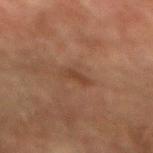The lesion was photographed on a routine skin check and not biopsied; there is no pathology result. Longest diameter approximately 3 mm. The lesion is located on the arm. A 15 mm close-up extracted from a 3D total-body photography capture. The lesion-visualizer software estimated a classifier nevus-likeness of about 0/100 and lesion-presence confidence of about 100/100. Imaged with cross-polarized lighting. A male subject aged 73–77.